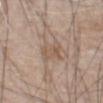Assessment: Recorded during total-body skin imaging; not selected for excision or biopsy. Background: The lesion is on the abdomen. A close-up tile cropped from a whole-body skin photograph, about 15 mm across. A male patient aged approximately 80.